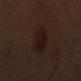Captured during whole-body skin photography for melanoma surveillance; the lesion was not biopsied.
A 15 mm crop from a total-body photograph taken for skin-cancer surveillance.
An algorithmic analysis of the crop reported an eccentricity of roughly 0.3 and a symmetry-axis asymmetry near 0.2. And it measured a border-irregularity index near 2.5/10, a within-lesion color-variation index near 2.5/10, and radial color variation of about 1.
Captured under cross-polarized illumination.
A male subject, aged around 50.
The lesion's longest dimension is about 3.5 mm.